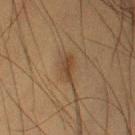  biopsy_status: not biopsied; imaged during a skin examination
  lesion_size:
    long_diameter_mm_approx: 3.0
  site: left upper arm
  lighting: cross-polarized
  patient:
    sex: male
    age_approx: 50
  image:
    source: total-body photography crop
    field_of_view_mm: 15